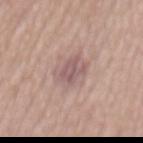biopsy_status: not biopsied; imaged during a skin examination
site: mid back
lighting: white-light
patient:
  sex: male
  age_approx: 55
lesion_size:
  long_diameter_mm_approx: 3.5
image:
  source: total-body photography crop
  field_of_view_mm: 15